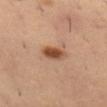Q: Was a biopsy performed?
A: total-body-photography surveillance lesion; no biopsy
Q: How was the tile lit?
A: cross-polarized illumination
Q: Patient demographics?
A: male, approximately 50 years of age
Q: What is the anatomic site?
A: the left thigh
Q: What is the lesion's diameter?
A: ≈3.5 mm
Q: How was this image acquired?
A: total-body-photography crop, ~15 mm field of view